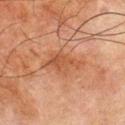Impression: The lesion was tiled from a total-body skin photograph and was not biopsied. Context: This is a cross-polarized tile. The lesion is located on the left thigh. The subject is a male aged around 80. Cropped from a total-body skin-imaging series; the visible field is about 15 mm. The total-body-photography lesion software estimated an area of roughly 8.5 mm², an outline eccentricity of about 0.8 (0 = round, 1 = elongated), and a shape-asymmetry score of about 0.35 (0 = symmetric). And it measured a detector confidence of about 100 out of 100 that the crop contains a lesion.